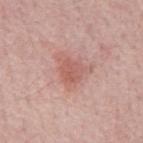Part of a total-body skin-imaging series; this lesion was reviewed on a skin check and was not flagged for biopsy. A region of skin cropped from a whole-body photographic capture, roughly 15 mm wide. Longest diameter approximately 2.5 mm. From the mid back. The lesion-visualizer software estimated an average lesion color of about L≈57 a*≈26 b*≈26 (CIELAB) and roughly 8 lightness units darker than nearby skin. The analysis additionally found a nevus-likeness score of about 40/100 and lesion-presence confidence of about 100/100. A male patient, roughly 65 years of age.